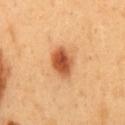This lesion was catalogued during total-body skin photography and was not selected for biopsy. The patient is a male about 50 years old. The lesion is located on the mid back. A roughly 15 mm field-of-view crop from a total-body skin photograph.